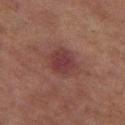{"biopsy_status": "not biopsied; imaged during a skin examination", "image": {"source": "total-body photography crop", "field_of_view_mm": 15}, "automated_metrics": {"eccentricity": 0.5, "shape_asymmetry": 0.2, "cielab_L": 33, "cielab_a": 22, "cielab_b": 18, "vs_skin_darker_L": 8.0, "vs_skin_contrast_norm": 7.5, "border_irregularity_0_10": 2.0, "color_variation_0_10": 3.0, "peripheral_color_asymmetry": 1.0, "nevus_likeness_0_100": 35, "lesion_detection_confidence_0_100": 100}, "site": "left thigh", "patient": {"sex": "female", "age_approx": 60}}